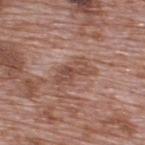workup: catalogued during a skin exam; not biopsied | patient: male, aged 68 to 72 | anatomic site: the upper back | size: about 4.5 mm | imaging modality: ~15 mm crop, total-body skin-cancer survey.